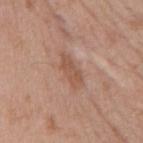Context:
A region of skin cropped from a whole-body photographic capture, roughly 15 mm wide. The subject is a male approximately 75 years of age. The lesion is located on the mid back. Imaged with white-light lighting.